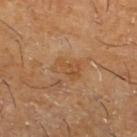| key | value |
|---|---|
| image source | ~15 mm tile from a whole-body skin photo |
| anatomic site | the left lower leg |
| patient | male, roughly 60 years of age |
| size | about 3 mm |
| lighting | cross-polarized |